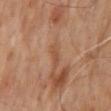biopsy status: total-body-photography surveillance lesion; no biopsy
image source: 15 mm crop, total-body photography
diameter: ~3 mm (longest diameter)
patient: male, in their mid-60s
illumination: cross-polarized illumination
anatomic site: the back
automated lesion analysis: an average lesion color of about L≈48 a*≈20 b*≈32 (CIELAB), roughly 6 lightness units darker than nearby skin, and a normalized lesion–skin contrast near 5.5; an automated nevus-likeness rating near 0 out of 100 and lesion-presence confidence of about 95/100